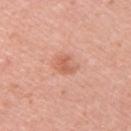Assessment:
The lesion was photographed on a routine skin check and not biopsied; there is no pathology result.
Image and clinical context:
A lesion tile, about 15 mm wide, cut from a 3D total-body photograph. A female subject, roughly 30 years of age. On the arm. Approximately 2.5 mm at its widest.